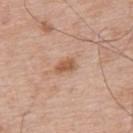Recorded during total-body skin imaging; not selected for excision or biopsy.
A male subject in their mid-60s.
The lesion is on the upper back.
Cropped from a total-body skin-imaging series; the visible field is about 15 mm.
Measured at roughly 2.5 mm in maximum diameter.
Imaged with white-light lighting.